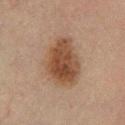{"image": {"source": "total-body photography crop", "field_of_view_mm": 15}, "site": "leg", "automated_metrics": {"area_mm2_approx": 21.0, "eccentricity": 0.7, "shape_asymmetry": 0.2, "border_irregularity_0_10": 2.0, "color_variation_0_10": 4.5, "peripheral_color_asymmetry": 1.5, "nevus_likeness_0_100": 100, "lesion_detection_confidence_0_100": 100}, "patient": {"sex": "male", "age_approx": 65}, "lesion_size": {"long_diameter_mm_approx": 6.0}, "lighting": "cross-polarized"}